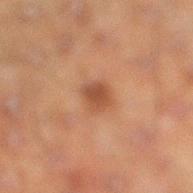Captured during whole-body skin photography for melanoma surveillance; the lesion was not biopsied. A 15 mm crop from a total-body photograph taken for skin-cancer surveillance. The tile uses cross-polarized illumination. The lesion is on the right lower leg. A male patient roughly 50 years of age.